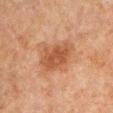workup = catalogued during a skin exam; not biopsied | body site = the left upper arm | tile lighting = cross-polarized illumination | acquisition = total-body-photography crop, ~15 mm field of view | image-analysis metrics = an eccentricity of roughly 0.65 and a symmetry-axis asymmetry near 0.2; a border-irregularity index near 2/10, a color-variation rating of about 2.5/10, and a peripheral color-asymmetry measure near 1; an automated nevus-likeness rating near 20 out of 100 and a lesion-detection confidence of about 100/100 | patient = male, aged 58 to 62.